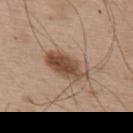Notes:
– notes — total-body-photography surveillance lesion; no biopsy
– illumination — white-light
– diameter — ~5.5 mm (longest diameter)
– site — the upper back
– imaging modality — 15 mm crop, total-body photography
– patient — male, approximately 55 years of age